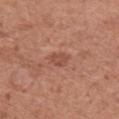This lesion was catalogued during total-body skin photography and was not selected for biopsy.
A female patient roughly 50 years of age.
The lesion is located on the chest.
A 15 mm close-up tile from a total-body photography series done for melanoma screening.